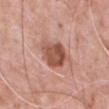The lesion was tiled from a total-body skin photograph and was not biopsied. Cropped from a total-body skin-imaging series; the visible field is about 15 mm. The lesion's longest dimension is about 4 mm. A male patient, aged 48–52. Automated image analysis of the tile measured a footprint of about 11 mm², an eccentricity of roughly 0.55, and a symmetry-axis asymmetry near 0.3. And it measured roughly 13 lightness units darker than nearby skin and a normalized border contrast of about 9. And it measured an automated nevus-likeness rating near 15 out of 100 and a detector confidence of about 100 out of 100 that the crop contains a lesion. The lesion is located on the chest.